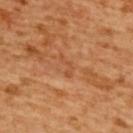Captured during whole-body skin photography for melanoma surveillance; the lesion was not biopsied.
Cropped from a total-body skin-imaging series; the visible field is about 15 mm.
Longest diameter approximately 3 mm.
The lesion is on the upper back.
A female subject aged around 55.
This is a cross-polarized tile.